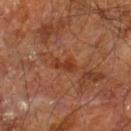follow-up: catalogued during a skin exam; not biopsied
lighting: cross-polarized illumination
diameter: ≈3 mm
image: ~15 mm crop, total-body skin-cancer survey
automated lesion analysis: an area of roughly 4 mm², an outline eccentricity of about 0.85 (0 = round, 1 = elongated), and a symmetry-axis asymmetry near 0.3
subject: male, aged 58–62
site: the leg